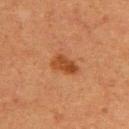<record>
<biopsy_status>not biopsied; imaged during a skin examination</biopsy_status>
<image>
  <source>total-body photography crop</source>
  <field_of_view_mm>15</field_of_view_mm>
</image>
<lighting>cross-polarized</lighting>
<lesion_size>
  <long_diameter_mm_approx>3.5</long_diameter_mm_approx>
</lesion_size>
<site>upper back</site>
<patient>
  <sex>female</sex>
  <age_approx>40</age_approx>
</patient>
<automated_metrics>
  <area_mm2_approx>5.5</area_mm2_approx>
  <eccentricity>0.85</eccentricity>
  <shape_asymmetry>0.25</shape_asymmetry>
  <cielab_L>39</cielab_L>
  <cielab_a>24</cielab_a>
  <cielab_b>34</cielab_b>
  <vs_skin_darker_L>9.0</vs_skin_darker_L>
  <vs_skin_contrast_norm>8.5</vs_skin_contrast_norm>
  <border_irregularity_0_10>2.5</border_irregularity_0_10>
  <color_variation_0_10>3.0</color_variation_0_10>
  <nevus_likeness_0_100>95</nevus_likeness_0_100>
  <lesion_detection_confidence_0_100>100</lesion_detection_confidence_0_100>
</automated_metrics>
</record>